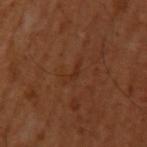The lesion was tiled from a total-body skin photograph and was not biopsied.
The patient is a male aged approximately 50.
This is a cross-polarized tile.
A close-up tile cropped from a whole-body skin photograph, about 15 mm across.
Approximately 3 mm at its widest.
Located on the left upper arm.